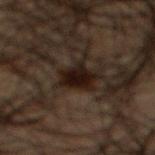Recorded during total-body skin imaging; not selected for excision or biopsy.